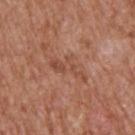A 15 mm close-up tile from a total-body photography series done for melanoma screening. On the upper back. The total-body-photography lesion software estimated a lesion color around L≈49 a*≈24 b*≈30 in CIELAB. The software also gave a color-variation rating of about 2/10 and radial color variation of about 0. It also reported a detector confidence of about 100 out of 100 that the crop contains a lesion. Approximately 5 mm at its widest. A male subject in their mid-60s.